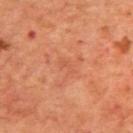This lesion was catalogued during total-body skin photography and was not selected for biopsy.
Captured under cross-polarized illumination.
A 15 mm crop from a total-body photograph taken for skin-cancer surveillance.
Located on the upper back.
Measured at roughly 2 mm in maximum diameter.
The lesion-visualizer software estimated an average lesion color of about L≈54 a*≈28 b*≈36 (CIELAB), about 4 CIELAB-L* units darker than the surrounding skin, and a normalized lesion–skin contrast near 3.
A male subject, in their 70s.